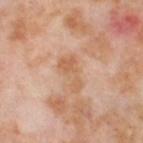<lesion>
  <biopsy_status>not biopsied; imaged during a skin examination</biopsy_status>
  <image>
    <source>total-body photography crop</source>
    <field_of_view_mm>15</field_of_view_mm>
  </image>
  <lighting>cross-polarized</lighting>
  <automated_metrics>
    <border_irregularity_0_10>7.0</border_irregularity_0_10>
    <color_variation_0_10>1.5</color_variation_0_10>
    <peripheral_color_asymmetry>0.5</peripheral_color_asymmetry>
    <nevus_likeness_0_100>0</nevus_likeness_0_100>
    <lesion_detection_confidence_0_100>100</lesion_detection_confidence_0_100>
  </automated_metrics>
  <site>leg</site>
  <patient>
    <sex>female</sex>
    <age_approx>55</age_approx>
  </patient>
</lesion>